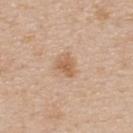The lesion was photographed on a routine skin check and not biopsied; there is no pathology result. An algorithmic analysis of the crop reported an area of roughly 4.5 mm², an outline eccentricity of about 0.65 (0 = round, 1 = elongated), and two-axis asymmetry of about 0.35. The analysis additionally found a border-irregularity index near 3.5/10, internal color variation of about 1.5 on a 0–10 scale, and radial color variation of about 0.5. This image is a 15 mm lesion crop taken from a total-body photograph. The tile uses white-light illumination. The lesion's longest dimension is about 2.5 mm. A female subject aged approximately 45. On the upper back.